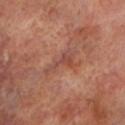A male patient, aged 68 to 72. Located on the left lower leg. Imaged with cross-polarized lighting. Automated tile analysis of the lesion measured an average lesion color of about L≈46 a*≈24 b*≈27 (CIELAB) and roughly 7 lightness units darker than nearby skin. The software also gave a border-irregularity index near 7/10 and radial color variation of about 1. The software also gave a nevus-likeness score of about 0/100 and lesion-presence confidence of about 85/100. A close-up tile cropped from a whole-body skin photograph, about 15 mm across.